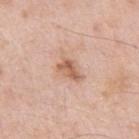{"biopsy_status": "not biopsied; imaged during a skin examination", "image": {"source": "total-body photography crop", "field_of_view_mm": 15}, "patient": {"sex": "male", "age_approx": 55}, "site": "left upper arm"}